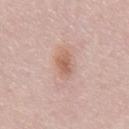Clinical impression:
Imaged during a routine full-body skin examination; the lesion was not biopsied and no histopathology is available.
Clinical summary:
Approximately 4 mm at its widest. This image is a 15 mm lesion crop taken from a total-body photograph. Automated image analysis of the tile measured a lesion area of about 6 mm² and two-axis asymmetry of about 0.2. And it measured a within-lesion color-variation index near 3/10 and radial color variation of about 1. This is a white-light tile. The subject is a male approximately 50 years of age. On the abdomen.